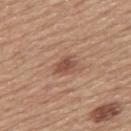Clinical impression: The lesion was photographed on a routine skin check and not biopsied; there is no pathology result. Image and clinical context: A 15 mm crop from a total-body photograph taken for skin-cancer surveillance. The patient is a female aged 53 to 57. The lesion is on the back.